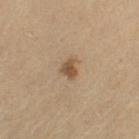{"biopsy_status": "not biopsied; imaged during a skin examination", "image": {"source": "total-body photography crop", "field_of_view_mm": 15}, "site": "right thigh", "lesion_size": {"long_diameter_mm_approx": 2.5}, "lighting": "cross-polarized", "patient": {"sex": "female", "age_approx": 70}}